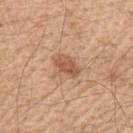follow-up: imaged on a skin check; not biopsied | lighting: white-light illumination | image-analysis metrics: a mean CIELAB color near L≈57 a*≈22 b*≈34, roughly 10 lightness units darker than nearby skin, and a lesion-to-skin contrast of about 7 (normalized; higher = more distinct); a border-irregularity index near 2.5/10, a within-lesion color-variation index near 3/10, and radial color variation of about 1 | patient: male, about 55 years old | acquisition: total-body-photography crop, ~15 mm field of view | site: the upper back.